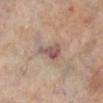Clinical impression:
No biopsy was performed on this lesion — it was imaged during a full skin examination and was not determined to be concerning.
Clinical summary:
This is a cross-polarized tile. A female subject about 60 years old. This image is a 15 mm lesion crop taken from a total-body photograph. Longest diameter approximately 3.5 mm. From the right lower leg. Automated tile analysis of the lesion measured a nevus-likeness score of about 0/100.